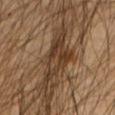<tbp_lesion>
  <biopsy_status>not biopsied; imaged during a skin examination</biopsy_status>
  <lighting>cross-polarized</lighting>
  <site>left forearm</site>
  <image>
    <source>total-body photography crop</source>
    <field_of_view_mm>15</field_of_view_mm>
  </image>
  <automated_metrics>
    <cielab_L>36</cielab_L>
    <cielab_a>15</cielab_a>
    <cielab_b>28</cielab_b>
    <vs_skin_darker_L>11.0</vs_skin_darker_L>
    <lesion_detection_confidence_0_100>0</lesion_detection_confidence_0_100>
  </automated_metrics>
  <lesion_size>
    <long_diameter_mm_approx>8.5</long_diameter_mm_approx>
  </lesion_size>
  <patient>
    <sex>male</sex>
    <age_approx>45</age_approx>
  </patient>
</tbp_lesion>